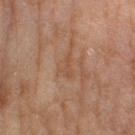• workup: no biopsy performed (imaged during a skin exam)
• site: the right lower leg
• lesion diameter: about 3 mm
• tile lighting: cross-polarized
• image-analysis metrics: a lesion area of about 3.5 mm², an outline eccentricity of about 0.85 (0 = round, 1 = elongated), and a symmetry-axis asymmetry near 0.6; a border-irregularity rating of about 6.5/10, internal color variation of about 0.5 on a 0–10 scale, and radial color variation of about 0
• image source: ~15 mm crop, total-body skin-cancer survey
• patient: female, about 60 years old